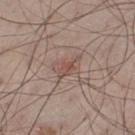{
  "biopsy_status": "not biopsied; imaged during a skin examination",
  "site": "left thigh",
  "automated_metrics": {
    "eccentricity": 0.9,
    "shape_asymmetry": 0.4,
    "border_irregularity_0_10": 5.0,
    "color_variation_0_10": 1.0
  },
  "patient": {
    "sex": "male",
    "age_approx": 60
  },
  "lighting": "white-light",
  "image": {
    "source": "total-body photography crop",
    "field_of_view_mm": 15
  },
  "lesion_size": {
    "long_diameter_mm_approx": 3.5
  }
}